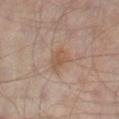Part of a total-body skin-imaging series; this lesion was reviewed on a skin check and was not flagged for biopsy.
A male subject, aged 63 to 67.
The tile uses cross-polarized illumination.
Longest diameter approximately 3 mm.
Cropped from a whole-body photographic skin survey; the tile spans about 15 mm.
The lesion is on the right thigh.